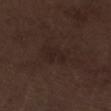Recorded during total-body skin imaging; not selected for excision or biopsy. A 15 mm close-up extracted from a 3D total-body photography capture. The lesion's longest dimension is about 3 mm. A male patient in their 70s. Located on the left lower leg. Imaged with white-light lighting.